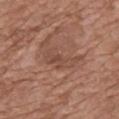This lesion was catalogued during total-body skin photography and was not selected for biopsy. Automated tile analysis of the lesion measured an area of roughly 7.5 mm² and a symmetry-axis asymmetry near 0.45. The analysis additionally found a border-irregularity index near 6.5/10, a within-lesion color-variation index near 2.5/10, and peripheral color asymmetry of about 1. The lesion is located on the mid back. The tile uses white-light illumination. Cropped from a whole-body photographic skin survey; the tile spans about 15 mm. A female subject, aged around 75.